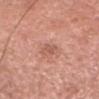• workup — imaged on a skin check; not biopsied
• patient — female, roughly 50 years of age
• automated lesion analysis — a lesion color around L≈57 a*≈25 b*≈29 in CIELAB, roughly 8 lightness units darker than nearby skin, and a normalized lesion–skin contrast near 5.5; an automated nevus-likeness rating near 0 out of 100
• diameter — ≈2.5 mm
• illumination — white-light
• location — the head or neck
• acquisition — total-body-photography crop, ~15 mm field of view